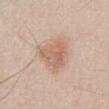Notes:
– biopsy status: total-body-photography surveillance lesion; no biopsy
– size: about 4.5 mm
– imaging modality: 15 mm crop, total-body photography
– illumination: white-light
– site: the abdomen
– patient: male, roughly 50 years of age